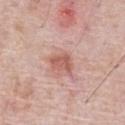{"biopsy_status": "not biopsied; imaged during a skin examination", "site": "chest", "patient": {"sex": "male", "age_approx": 70}, "automated_metrics": {"cielab_L": 58, "cielab_a": 25, "cielab_b": 27, "vs_skin_darker_L": 10.0, "nevus_likeness_0_100": 85, "lesion_detection_confidence_0_100": 100}, "image": {"source": "total-body photography crop", "field_of_view_mm": 15}, "lesion_size": {"long_diameter_mm_approx": 3.0}}